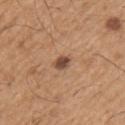Q: Was this lesion biopsied?
A: total-body-photography surveillance lesion; no biopsy
Q: Where on the body is the lesion?
A: the left upper arm
Q: Patient demographics?
A: male, roughly 65 years of age
Q: Lesion size?
A: ≈3 mm
Q: How was this image acquired?
A: ~15 mm crop, total-body skin-cancer survey
Q: How was the tile lit?
A: white-light illumination